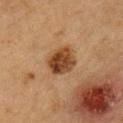Impression:
Recorded during total-body skin imaging; not selected for excision or biopsy.
Background:
A close-up tile cropped from a whole-body skin photograph, about 15 mm across. Automated tile analysis of the lesion measured a footprint of about 9.5 mm² and an outline eccentricity of about 0.55 (0 = round, 1 = elongated). The software also gave a border-irregularity rating of about 2/10 and a color-variation rating of about 5.5/10. On the chest. The lesion's longest dimension is about 4 mm. This is a cross-polarized tile. A male subject, aged 58 to 62.